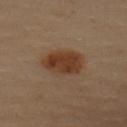Approximately 5 mm at its widest. A female patient, in their 60s. The lesion is located on the upper back. The lesion-visualizer software estimated an average lesion color of about L≈32 a*≈17 b*≈26 (CIELAB), roughly 9 lightness units darker than nearby skin, and a normalized border contrast of about 9.5. It also reported a border-irregularity rating of about 1.5/10, a within-lesion color-variation index near 3/10, and radial color variation of about 1. And it measured a nevus-likeness score of about 100/100 and a lesion-detection confidence of about 100/100. Cropped from a total-body skin-imaging series; the visible field is about 15 mm. The tile uses cross-polarized illumination.